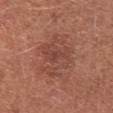The lesion was photographed on a routine skin check and not biopsied; there is no pathology result.
Measured at roughly 5.5 mm in maximum diameter.
A roughly 15 mm field-of-view crop from a total-body skin photograph.
The lesion-visualizer software estimated an outline eccentricity of about 0.45 (0 = round, 1 = elongated) and two-axis asymmetry of about 0.35. It also reported a lesion color around L≈45 a*≈23 b*≈28 in CIELAB, about 7 CIELAB-L* units darker than the surrounding skin, and a normalized lesion–skin contrast near 5. It also reported an automated nevus-likeness rating near 0 out of 100.
A female patient, about 50 years old.
From the upper back.
Captured under white-light illumination.